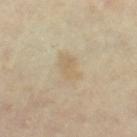Impression:
Part of a total-body skin-imaging series; this lesion was reviewed on a skin check and was not flagged for biopsy.
Clinical summary:
A female subject approximately 45 years of age. A close-up tile cropped from a whole-body skin photograph, about 15 mm across. Approximately 3 mm at its widest. Automated tile analysis of the lesion measured a footprint of about 5.5 mm², an eccentricity of roughly 0.75, and a shape-asymmetry score of about 0.25 (0 = symmetric). And it measured a lesion color around L≈64 a*≈10 b*≈33 in CIELAB, roughly 6 lightness units darker than nearby skin, and a normalized border contrast of about 4.5. The analysis additionally found a nevus-likeness score of about 5/100. The tile uses cross-polarized illumination. The lesion is on the left thigh.